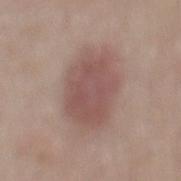site: the mid back
TBP lesion metrics: an automated nevus-likeness rating near 100 out of 100 and lesion-presence confidence of about 100/100
patient: male, aged approximately 70
lighting: white-light illumination
acquisition: total-body-photography crop, ~15 mm field of view